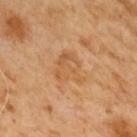A male subject in their mid-60s. This image is a 15 mm lesion crop taken from a total-body photograph. Longest diameter approximately 4 mm. Imaged with cross-polarized lighting.